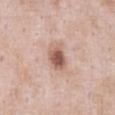biopsy status — catalogued during a skin exam; not biopsied
site — the chest
imaging modality — ~15 mm tile from a whole-body skin photo
lighting — white-light
automated lesion analysis — a footprint of about 7 mm²
patient — male, in their mid-50s